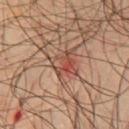Captured during whole-body skin photography for melanoma surveillance; the lesion was not biopsied.
The patient is a male approximately 65 years of age.
From the chest.
A 15 mm close-up tile from a total-body photography series done for melanoma screening.
This is a cross-polarized tile.
Automated image analysis of the tile measured an average lesion color of about L≈48 a*≈24 b*≈29 (CIELAB) and about 9 CIELAB-L* units darker than the surrounding skin.